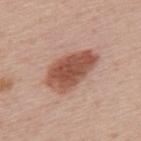Q: Was a biopsy performed?
A: no biopsy performed (imaged during a skin exam)
Q: What is the anatomic site?
A: the upper back
Q: Illumination type?
A: white-light
Q: How large is the lesion?
A: ~6.5 mm (longest diameter)
Q: How was this image acquired?
A: ~15 mm tile from a whole-body skin photo
Q: Automated lesion metrics?
A: an area of roughly 18 mm² and a shape-asymmetry score of about 0.2 (0 = symmetric); a color-variation rating of about 4/10 and a peripheral color-asymmetry measure near 1
Q: Who is the patient?
A: female, aged around 60